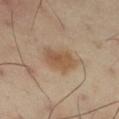{
  "biopsy_status": "not biopsied; imaged during a skin examination",
  "lesion_size": {
    "long_diameter_mm_approx": 4.0
  },
  "image": {
    "source": "total-body photography crop",
    "field_of_view_mm": 15
  },
  "patient": {
    "sex": "male",
    "age_approx": 55
  },
  "lighting": "cross-polarized",
  "site": "right thigh"
}